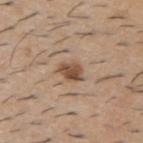- follow-up · no biopsy performed (imaged during a skin exam)
- TBP lesion metrics · an area of roughly 5.5 mm² and two-axis asymmetry of about 0.2
- illumination · white-light illumination
- lesion diameter · ~3 mm (longest diameter)
- anatomic site · the upper back
- patient · male, aged 58 to 62
- imaging modality · ~15 mm crop, total-body skin-cancer survey